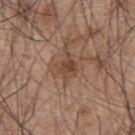Q: Is there a histopathology result?
A: catalogued during a skin exam; not biopsied
Q: What is the imaging modality?
A: ~15 mm crop, total-body skin-cancer survey
Q: Where on the body is the lesion?
A: the back
Q: How was the tile lit?
A: white-light
Q: What did automated image analysis measure?
A: an automated nevus-likeness rating near 10 out of 100
Q: What is the lesion's diameter?
A: ≈2.5 mm
Q: Patient demographics?
A: male, in their mid- to late 40s